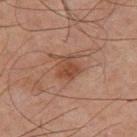Q: Was a biopsy performed?
A: imaged on a skin check; not biopsied
Q: Patient demographics?
A: male, approximately 65 years of age
Q: What is the imaging modality?
A: ~15 mm tile from a whole-body skin photo
Q: What is the anatomic site?
A: the right thigh
Q: Illumination type?
A: cross-polarized